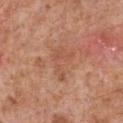Findings:
– workup · catalogued during a skin exam; not biopsied
– lesion size · about 4 mm
– patient · male, aged approximately 65
– acquisition · 15 mm crop, total-body photography
– location · the chest